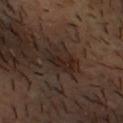Findings:
* follow-up — catalogued during a skin exam; not biopsied
* illumination — cross-polarized illumination
* subject — male, roughly 40 years of age
* image source — ~15 mm tile from a whole-body skin photo
* automated lesion analysis — a lesion area of about 8.5 mm², an outline eccentricity of about 0.85 (0 = round, 1 = elongated), and two-axis asymmetry of about 0.3; a border-irregularity index near 4/10, internal color variation of about 4 on a 0–10 scale, and a peripheral color-asymmetry measure near 1.5
* anatomic site — the head or neck